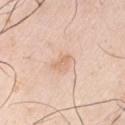{"image": {"source": "total-body photography crop", "field_of_view_mm": 15}, "patient": {"sex": "male", "age_approx": 40}, "site": "right upper arm"}